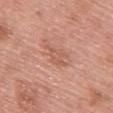biopsy_status: not biopsied; imaged during a skin examination
lesion_size:
  long_diameter_mm_approx: 3.5
site: back
image:
  source: total-body photography crop
  field_of_view_mm: 15
patient:
  sex: female
  age_approx: 60
lighting: white-light